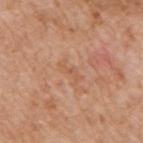Clinical impression: Recorded during total-body skin imaging; not selected for excision or biopsy. Background: A male subject, approximately 60 years of age. Measured at roughly 3 mm in maximum diameter. The lesion is on the right upper arm. A close-up tile cropped from a whole-body skin photograph, about 15 mm across.